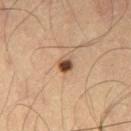Impression:
The lesion was photographed on a routine skin check and not biopsied; there is no pathology result.
Acquisition and patient details:
A roughly 15 mm field-of-view crop from a total-body skin photograph. On the left thigh. The subject is a male aged approximately 65. Measured at roughly 2 mm in maximum diameter. This is a cross-polarized tile.